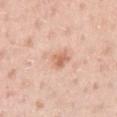Q: Is there a histopathology result?
A: no biopsy performed (imaged during a skin exam)
Q: What kind of image is this?
A: total-body-photography crop, ~15 mm field of view
Q: Lesion size?
A: about 3.5 mm
Q: Lesion location?
A: the left upper arm
Q: Who is the patient?
A: male, about 40 years old
Q: Automated lesion metrics?
A: border irregularity of about 3.5 on a 0–10 scale and internal color variation of about 5.5 on a 0–10 scale
Q: How was the tile lit?
A: white-light